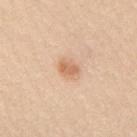Case summary:
• notes — total-body-photography surveillance lesion; no biopsy
• size — ~2.5 mm (longest diameter)
• subject — female, in their 50s
• acquisition — ~15 mm crop, total-body skin-cancer survey
• tile lighting — white-light illumination
• automated metrics — a classifier nevus-likeness of about 90/100 and a detector confidence of about 100 out of 100 that the crop contains a lesion
• location — the back